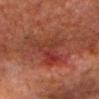Findings:
* notes · no biopsy performed (imaged during a skin exam)
* lesion diameter · ~5 mm (longest diameter)
* body site · the head or neck
* illumination · cross-polarized illumination
* subject · male, aged 78 to 82
* automated metrics · a lesion color around L≈29 a*≈24 b*≈24 in CIELAB, roughly 6 lightness units darker than nearby skin, and a normalized lesion–skin contrast near 6; a border-irregularity index near 6.5/10 and a within-lesion color-variation index near 7/10; a nevus-likeness score of about 0/100 and lesion-presence confidence of about 95/100
* imaging modality · 15 mm crop, total-body photography